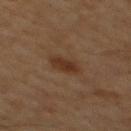| feature | finding |
|---|---|
| follow-up | catalogued during a skin exam; not biopsied |
| patient | male, roughly 60 years of age |
| acquisition | total-body-photography crop, ~15 mm field of view |
| automated metrics | a nevus-likeness score of about 80/100 and a detector confidence of about 100 out of 100 that the crop contains a lesion |
| site | the mid back |